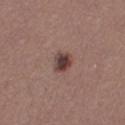Impression: The lesion was photographed on a routine skin check and not biopsied; there is no pathology result. Acquisition and patient details: Located on the right thigh. Approximately 2.5 mm at its widest. A female patient, aged around 30. Automated tile analysis of the lesion measured a mean CIELAB color near L≈40 a*≈18 b*≈20, roughly 13 lightness units darker than nearby skin, and a normalized border contrast of about 10.5. This is a white-light tile. A 15 mm close-up tile from a total-body photography series done for melanoma screening.